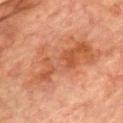Clinical impression: This lesion was catalogued during total-body skin photography and was not selected for biopsy. Context: Captured under cross-polarized illumination. From the front of the torso. A male patient, about 75 years old. This image is a 15 mm lesion crop taken from a total-body photograph.